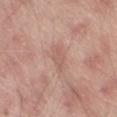Q: What is the imaging modality?
A: total-body-photography crop, ~15 mm field of view
Q: How large is the lesion?
A: about 3.5 mm
Q: Where on the body is the lesion?
A: the left thigh
Q: Illumination type?
A: white-light
Q: What are the patient's age and sex?
A: male, in their mid-60s